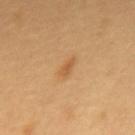workup: no biopsy performed (imaged during a skin exam)
image: ~15 mm tile from a whole-body skin photo
subject: female, aged approximately 60
lesion size: ~2.5 mm (longest diameter)
anatomic site: the back
tile lighting: cross-polarized illumination